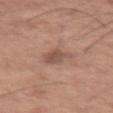Notes:
* follow-up · catalogued during a skin exam; not biopsied
* imaging modality · ~15 mm tile from a whole-body skin photo
* image-analysis metrics · a lesion area of about 5 mm² and an outline eccentricity of about 0.65 (0 = round, 1 = elongated); an average lesion color of about L≈51 a*≈20 b*≈25 (CIELAB), a lesion–skin lightness drop of about 9, and a normalized border contrast of about 6.5; a nevus-likeness score of about 20/100 and a detector confidence of about 100 out of 100 that the crop contains a lesion
* site · the left thigh
* subject · male, in their mid-60s
* lesion size · about 3 mm
* lighting · white-light illumination